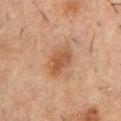workup: total-body-photography surveillance lesion; no biopsy
patient: male, about 50 years old
lesion diameter: about 4 mm
body site: the chest
automated lesion analysis: a border-irregularity index near 2/10 and internal color variation of about 3 on a 0–10 scale
tile lighting: cross-polarized
image source: 15 mm crop, total-body photography